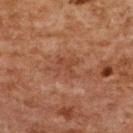Impression: The lesion was photographed on a routine skin check and not biopsied; there is no pathology result. Context: A female subject aged 58–62. Cropped from a total-body skin-imaging series; the visible field is about 15 mm. The lesion is on the back. The lesion's longest dimension is about 3 mm. Imaged with cross-polarized lighting.